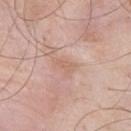Imaged during a routine full-body skin examination; the lesion was not biopsied and no histopathology is available.
A male subject roughly 55 years of age.
A 15 mm close-up tile from a total-body photography series done for melanoma screening.
Imaged with white-light lighting.
Approximately 3 mm at its widest.
Automated tile analysis of the lesion measured an area of roughly 3 mm². It also reported a nevus-likeness score of about 0/100 and a lesion-detection confidence of about 100/100.
Located on the chest.